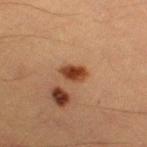| key | value |
|---|---|
| workup | total-body-photography surveillance lesion; no biopsy |
| anatomic site | the right thigh |
| subject | male, roughly 40 years of age |
| image source | ~15 mm tile from a whole-body skin photo |